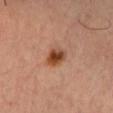Acquisition and patient details:
The lesion-visualizer software estimated a lesion area of about 6 mm², an eccentricity of roughly 0.7, and a shape-asymmetry score of about 0.25 (0 = symmetric). It also reported a border-irregularity rating of about 2.5/10, a color-variation rating of about 6.5/10, and peripheral color asymmetry of about 2. The lesion is located on the chest. A region of skin cropped from a whole-body photographic capture, roughly 15 mm wide. A male patient, aged 18–22. About 3.5 mm across. This is a cross-polarized tile.